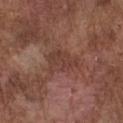| key | value |
|---|---|
| biopsy status | imaged on a skin check; not biopsied |
| size | ~4 mm (longest diameter) |
| anatomic site | the chest |
| image-analysis metrics | a lesion area of about 9 mm² and two-axis asymmetry of about 0.4 |
| lighting | white-light |
| patient | male, approximately 75 years of age |
| image | ~15 mm crop, total-body skin-cancer survey |